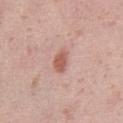Impression:
No biopsy was performed on this lesion — it was imaged during a full skin examination and was not determined to be concerning.
Background:
The recorded lesion diameter is about 2.5 mm. Cropped from a whole-body photographic skin survey; the tile spans about 15 mm. The lesion-visualizer software estimated border irregularity of about 2 on a 0–10 scale. A female subject in their 40s. Captured under white-light illumination. On the right thigh.